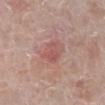The lesion was photographed on a routine skin check and not biopsied; there is no pathology result.
This is a white-light tile.
A female subject aged approximately 70.
About 3 mm across.
A close-up tile cropped from a whole-body skin photograph, about 15 mm across.
On the right lower leg.
An algorithmic analysis of the crop reported a border-irregularity index near 2.5/10 and a within-lesion color-variation index near 2.5/10. And it measured a nevus-likeness score of about 15/100 and lesion-presence confidence of about 100/100.